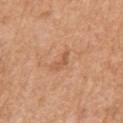notes = catalogued during a skin exam; not biopsied | patient = male, approximately 70 years of age | lighting = white-light | site = the left upper arm | image = ~15 mm tile from a whole-body skin photo.